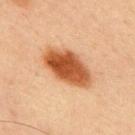workup = catalogued during a skin exam; not biopsied | illumination = cross-polarized | body site = the chest | subject = male, aged 68–72 | size = about 6.5 mm | image = total-body-photography crop, ~15 mm field of view | TBP lesion metrics = a color-variation rating of about 5/10 and radial color variation of about 1.5.